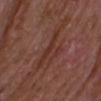Q: Automated lesion metrics?
A: a lesion area of about 8.5 mm² and an outline eccentricity of about 0.9 (0 = round, 1 = elongated)
Q: Illumination type?
A: white-light
Q: What is the lesion's diameter?
A: about 6 mm
Q: What is the imaging modality?
A: 15 mm crop, total-body photography
Q: Who is the patient?
A: male, in their mid-60s
Q: Where on the body is the lesion?
A: the upper back
Q: What is the histopathologic diagnosis?
A: a lichen planus-like keratosis — a benign lesion